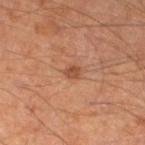{"biopsy_status": "not biopsied; imaged during a skin examination"}